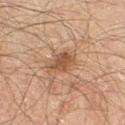The lesion was photographed on a routine skin check and not biopsied; there is no pathology result.
The patient is a male roughly 60 years of age.
Cropped from a total-body skin-imaging series; the visible field is about 15 mm.
Located on the left thigh.
Imaged with cross-polarized lighting.